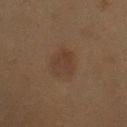lesion size: ~3.5 mm (longest diameter); acquisition: ~15 mm crop, total-body skin-cancer survey; subject: female, in their 50s; body site: the left upper arm; illumination: cross-polarized.